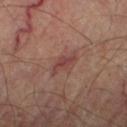{"biopsy_status": "not biopsied; imaged during a skin examination", "lesion_size": {"long_diameter_mm_approx": 3.5}, "automated_metrics": {"area_mm2_approx": 4.0, "eccentricity": 0.9, "shape_asymmetry": 0.35, "vs_skin_darker_L": 8.0, "vs_skin_contrast_norm": 6.5}, "image": {"source": "total-body photography crop", "field_of_view_mm": 15}, "site": "right thigh", "patient": {"sex": "male", "age_approx": 70}}